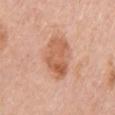{"biopsy_status": "not biopsied; imaged during a skin examination", "site": "arm", "lighting": "white-light", "patient": {"sex": "female", "age_approx": 65}, "lesion_size": {"long_diameter_mm_approx": 5.0}, "image": {"source": "total-body photography crop", "field_of_view_mm": 15}}